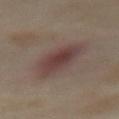Recorded during total-body skin imaging; not selected for excision or biopsy.
From the abdomen.
A female patient, aged around 55.
Automated tile analysis of the lesion measured a footprint of about 13 mm², an outline eccentricity of about 0.8 (0 = round, 1 = elongated), and a symmetry-axis asymmetry near 0.25. It also reported a normalized border contrast of about 8. The software also gave a classifier nevus-likeness of about 90/100 and a detector confidence of about 100 out of 100 that the crop contains a lesion.
A 15 mm close-up extracted from a 3D total-body photography capture.